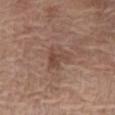notes: catalogued during a skin exam; not biopsied | image: 15 mm crop, total-body photography | site: the chest | patient: female, approximately 80 years of age | tile lighting: white-light illumination | lesion diameter: about 3 mm.